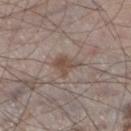No biopsy was performed on this lesion — it was imaged during a full skin examination and was not determined to be concerning. A region of skin cropped from a whole-body photographic capture, roughly 15 mm wide. On the left lower leg. A male patient aged approximately 60. This is a white-light tile.